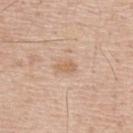Q: Is there a histopathology result?
A: catalogued during a skin exam; not biopsied
Q: Patient demographics?
A: male, in their mid-50s
Q: What kind of image is this?
A: total-body-photography crop, ~15 mm field of view
Q: What did automated image analysis measure?
A: a lesion area of about 3.5 mm², an eccentricity of roughly 0.75, and a symmetry-axis asymmetry near 0.25; an average lesion color of about L≈64 a*≈18 b*≈33 (CIELAB), roughly 8 lightness units darker than nearby skin, and a normalized border contrast of about 5.5; an automated nevus-likeness rating near 0 out of 100 and a lesion-detection confidence of about 100/100
Q: What lighting was used for the tile?
A: white-light illumination
Q: Where on the body is the lesion?
A: the upper back
Q: Lesion size?
A: ~2.5 mm (longest diameter)